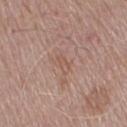Findings:
* illumination · white-light illumination
* anatomic site · the chest
* image · ~15 mm tile from a whole-body skin photo
* patient · male, aged 73 to 77